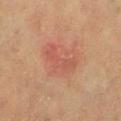This lesion was catalogued during total-body skin photography and was not selected for biopsy.
This image is a 15 mm lesion crop taken from a total-body photograph.
About 4.5 mm across.
The patient is a female aged around 55.
From the leg.
Imaged with cross-polarized lighting.